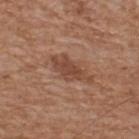Image and clinical context:
A 15 mm close-up tile from a total-body photography series done for melanoma screening. A male subject, aged approximately 65. On the upper back. The tile uses white-light illumination. The lesion's longest dimension is about 5 mm.